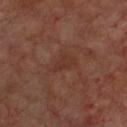Captured during whole-body skin photography for melanoma surveillance; the lesion was not biopsied.
The tile uses cross-polarized illumination.
The lesion-visualizer software estimated a lesion color around L≈32 a*≈20 b*≈24 in CIELAB.
Cropped from a whole-body photographic skin survey; the tile spans about 15 mm.
The lesion is located on the chest.
The patient is a male aged around 70.